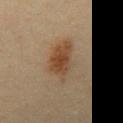{"biopsy_status": "not biopsied; imaged during a skin examination", "automated_metrics": {"border_irregularity_0_10": 3.5, "peripheral_color_asymmetry": 1.5, "nevus_likeness_0_100": 95}, "lighting": "cross-polarized", "patient": {"sex": "male", "age_approx": 35}, "image": {"source": "total-body photography crop", "field_of_view_mm": 15}, "site": "back", "lesion_size": {"long_diameter_mm_approx": 6.0}}